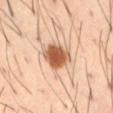<tbp_lesion>
<biopsy_status>not biopsied; imaged during a skin examination</biopsy_status>
<image>
  <source>total-body photography crop</source>
  <field_of_view_mm>15</field_of_view_mm>
</image>
<patient>
  <sex>male</sex>
  <age_approx>40</age_approx>
</patient>
<site>abdomen</site>
<lesion_size>
  <long_diameter_mm_approx>3.5</long_diameter_mm_approx>
</lesion_size>
</tbp_lesion>